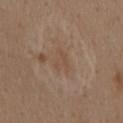{
  "patient": {
    "sex": "female",
    "age_approx": 40
  },
  "site": "mid back",
  "automated_metrics": {
    "border_irregularity_0_10": 5.0,
    "color_variation_0_10": 1.5,
    "nevus_likeness_0_100": 0,
    "lesion_detection_confidence_0_100": 100
  },
  "image": {
    "source": "total-body photography crop",
    "field_of_view_mm": 15
  },
  "lighting": "white-light"
}